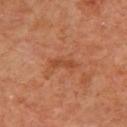| feature | finding |
|---|---|
| notes | catalogued during a skin exam; not biopsied |
| subject | female, approximately 40 years of age |
| image source | ~15 mm tile from a whole-body skin photo |
| lighting | cross-polarized illumination |
| diameter | about 3 mm |
| automated metrics | a lesion area of about 2.5 mm² and an outline eccentricity of about 0.95 (0 = round, 1 = elongated); a mean CIELAB color near L≈47 a*≈28 b*≈37 and a normalized border contrast of about 6; a border-irregularity rating of about 3.5/10 and peripheral color asymmetry of about 0; an automated nevus-likeness rating near 0 out of 100 and a lesion-detection confidence of about 100/100 |
| site | the upper back |